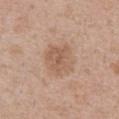workup: no biopsy performed (imaged during a skin exam) | image: 15 mm crop, total-body photography | subject: male, about 55 years old | illumination: white-light illumination | image-analysis metrics: a lesion area of about 14 mm² and an outline eccentricity of about 0.6 (0 = round, 1 = elongated); a border-irregularity rating of about 1.5/10 and radial color variation of about 1 | location: the chest.